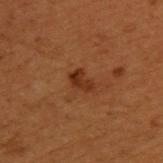Captured during whole-body skin photography for melanoma surveillance; the lesion was not biopsied.
An algorithmic analysis of the crop reported an area of roughly 3.5 mm², an outline eccentricity of about 0.8 (0 = round, 1 = elongated), and a symmetry-axis asymmetry near 0.4. The software also gave a border-irregularity index near 4/10, a within-lesion color-variation index near 2.5/10, and peripheral color asymmetry of about 1.
A 15 mm crop from a total-body photograph taken for skin-cancer surveillance.
The tile uses cross-polarized illumination.
From the upper back.
A male patient, aged 48–52.